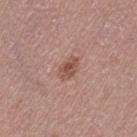biopsy status: imaged on a skin check; not biopsied | diameter: ~3 mm (longest diameter) | subject: female, aged around 50 | image source: 15 mm crop, total-body photography | location: the left thigh | lighting: white-light.